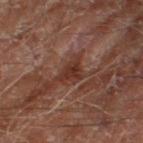{"biopsy_status": "not biopsied; imaged during a skin examination", "site": "right thigh", "lesion_size": {"long_diameter_mm_approx": 3.5}, "lighting": "cross-polarized", "patient": {"sex": "male", "age_approx": 70}, "image": {"source": "total-body photography crop", "field_of_view_mm": 15}}